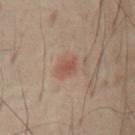Case summary:
- biopsy status: no biopsy performed (imaged during a skin exam)
- tile lighting: cross-polarized
- anatomic site: the front of the torso
- imaging modality: 15 mm crop, total-body photography
- patient: female, aged 53 to 57
- lesion diameter: ≈2.5 mm